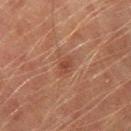Captured during whole-body skin photography for melanoma surveillance; the lesion was not biopsied. The subject is a male about 75 years old. The lesion is located on the right thigh. A region of skin cropped from a whole-body photographic capture, roughly 15 mm wide. The tile uses cross-polarized illumination.